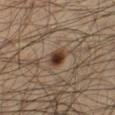Impression: Part of a total-body skin-imaging series; this lesion was reviewed on a skin check and was not flagged for biopsy. Background: This is a cross-polarized tile. Longest diameter approximately 2.5 mm. The lesion-visualizer software estimated a lesion area of about 4 mm². The software also gave an average lesion color of about L≈33 a*≈16 b*≈25 (CIELAB), about 13 CIELAB-L* units darker than the surrounding skin, and a normalized border contrast of about 12. The patient is a male approximately 35 years of age. The lesion is located on the leg. A close-up tile cropped from a whole-body skin photograph, about 15 mm across.